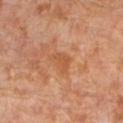Notes:
- workup: imaged on a skin check; not biopsied
- illumination: cross-polarized illumination
- lesion size: about 3 mm
- anatomic site: the left lower leg
- image-analysis metrics: a color-variation rating of about 2/10 and radial color variation of about 1; a detector confidence of about 100 out of 100 that the crop contains a lesion
- patient: male, approximately 30 years of age
- image source: ~15 mm tile from a whole-body skin photo